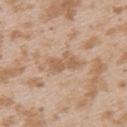follow-up: imaged on a skin check; not biopsied | subject: female, roughly 25 years of age | lesion size: ~4 mm (longest diameter) | tile lighting: white-light | site: the upper back | image-analysis metrics: a lesion area of about 6 mm² and an outline eccentricity of about 0.85 (0 = round, 1 = elongated); an average lesion color of about L≈59 a*≈18 b*≈32 (CIELAB) and a normalized border contrast of about 6; a nevus-likeness score of about 0/100 and a detector confidence of about 95 out of 100 that the crop contains a lesion | image source: ~15 mm tile from a whole-body skin photo.